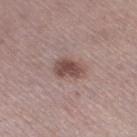Impression:
The lesion was photographed on a routine skin check and not biopsied; there is no pathology result.
Acquisition and patient details:
A female subject roughly 60 years of age. Located on the right thigh. A close-up tile cropped from a whole-body skin photograph, about 15 mm across.